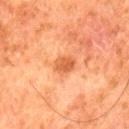Part of a total-body skin-imaging series; this lesion was reviewed on a skin check and was not flagged for biopsy. The tile uses cross-polarized illumination. The lesion-visualizer software estimated a lesion area of about 5 mm². It also reported a lesion color around L≈48 a*≈26 b*≈36 in CIELAB and a normalized lesion–skin contrast near 7. The analysis additionally found a border-irregularity index near 1.5/10 and a within-lesion color-variation index near 2.5/10. It also reported lesion-presence confidence of about 100/100. A male patient, approximately 80 years of age. A 15 mm close-up extracted from a 3D total-body photography capture. Located on the left thigh.